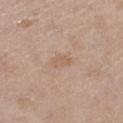follow-up — no biopsy performed (imaged during a skin exam)
acquisition — ~15 mm tile from a whole-body skin photo
tile lighting — white-light
lesion size — ≈2.5 mm
subject — female, in their 40s
site — the leg